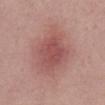workup: total-body-photography surveillance lesion; no biopsy
illumination: white-light illumination
acquisition: total-body-photography crop, ~15 mm field of view
body site: the abdomen
patient: male, in their mid- to late 50s
size: ~5 mm (longest diameter)